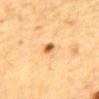Captured during whole-body skin photography for melanoma surveillance; the lesion was not biopsied. Automated tile analysis of the lesion measured a shape eccentricity near 0.7 and a symmetry-axis asymmetry near 0.3. This image is a 15 mm lesion crop taken from a total-body photograph. Longest diameter approximately 2.5 mm. A male patient aged approximately 85 or older. This is a cross-polarized tile. On the abdomen.